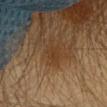notes: catalogued during a skin exam; not biopsied
lighting: cross-polarized
image source: ~15 mm crop, total-body skin-cancer survey
subject: female, aged around 20
body site: the head or neck
diameter: ~2.5 mm (longest diameter)